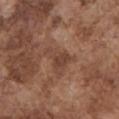| key | value |
|---|---|
| notes | catalogued during a skin exam; not biopsied |
| lighting | white-light |
| site | the chest |
| image source | ~15 mm tile from a whole-body skin photo |
| patient | male, aged around 75 |
| automated metrics | a lesion–skin lightness drop of about 7; a border-irregularity index near 6/10, a within-lesion color-variation index near 2.5/10, and peripheral color asymmetry of about 1 |
| size | ~4 mm (longest diameter) |